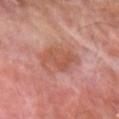* follow-up: catalogued during a skin exam; not biopsied